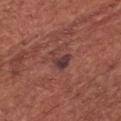Located on the head or neck. This is a white-light tile. A roughly 15 mm field-of-view crop from a total-body skin photograph. Automated tile analysis of the lesion measured a lesion area of about 4 mm², a shape eccentricity near 0.75, and a shape-asymmetry score of about 0.5 (0 = symmetric). The recorded lesion diameter is about 3 mm. The patient is a male aged approximately 65.